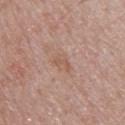- biopsy status — total-body-photography surveillance lesion; no biopsy
- TBP lesion metrics — a footprint of about 4 mm², a shape eccentricity near 0.65, and two-axis asymmetry of about 0.35; an automated nevus-likeness rating near 0 out of 100 and a detector confidence of about 100 out of 100 that the crop contains a lesion
- body site — the upper back
- tile lighting — white-light illumination
- patient — male, aged 48–52
- image source — ~15 mm crop, total-body skin-cancer survey
- size — ≈2.5 mm